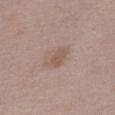<case>
<biopsy_status>not biopsied; imaged during a skin examination</biopsy_status>
<automated_metrics>
  <area_mm2_approx>5.0</area_mm2_approx>
  <eccentricity>0.8</eccentricity>
  <shape_asymmetry>0.35</shape_asymmetry>
  <border_irregularity_0_10>4.0</border_irregularity_0_10>
  <nevus_likeness_0_100>10</nevus_likeness_0_100>
  <lesion_detection_confidence_0_100>100</lesion_detection_confidence_0_100>
</automated_metrics>
<image>
  <source>total-body photography crop</source>
  <field_of_view_mm>15</field_of_view_mm>
</image>
<site>right thigh</site>
<lighting>white-light</lighting>
<patient>
  <sex>female</sex>
  <age_approx>50</age_approx>
</patient>
</case>